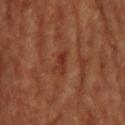Recorded during total-body skin imaging; not selected for excision or biopsy. A 15 mm crop from a total-body photograph taken for skin-cancer surveillance. The lesion is located on the head or neck.